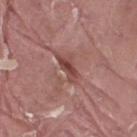Notes:
* anatomic site · the right thigh
* diameter · about 3.5 mm
* patient · male, roughly 40 years of age
* TBP lesion metrics · a border-irregularity index near 4.5/10, internal color variation of about 2 on a 0–10 scale, and peripheral color asymmetry of about 0.5
* imaging modality · ~15 mm tile from a whole-body skin photo